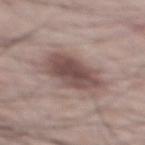This lesion was catalogued during total-body skin photography and was not selected for biopsy. Automated image analysis of the tile measured an outline eccentricity of about 0.75 (0 = round, 1 = elongated) and a symmetry-axis asymmetry near 0.2. The analysis additionally found an automated nevus-likeness rating near 45 out of 100 and a lesion-detection confidence of about 100/100. Cropped from a whole-body photographic skin survey; the tile spans about 15 mm. The lesion is located on the mid back. A male subject in their mid- to late 60s. Measured at roughly 5.5 mm in maximum diameter.